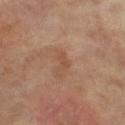{
  "biopsy_status": "not biopsied; imaged during a skin examination",
  "patient": {
    "sex": "female",
    "age_approx": 65
  },
  "site": "left leg",
  "image": {
    "source": "total-body photography crop",
    "field_of_view_mm": 15
  },
  "lesion_size": {
    "long_diameter_mm_approx": 3.0
  },
  "lighting": "cross-polarized"
}